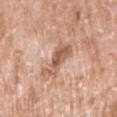Assessment: This lesion was catalogued during total-body skin photography and was not selected for biopsy. Clinical summary: Imaged with white-light lighting. The lesion is on the right upper arm. The lesion's longest dimension is about 5 mm. A 15 mm close-up extracted from a 3D total-body photography capture. A female subject aged 83 or over.